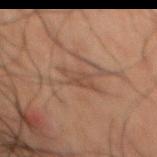biopsy status = catalogued during a skin exam; not biopsied | patient = male, aged 48 to 52 | imaging modality = 15 mm crop, total-body photography | body site = the abdomen | size = ~3 mm (longest diameter).